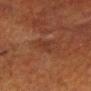Image and clinical context: Automated tile analysis of the lesion measured an area of roughly 3 mm², an outline eccentricity of about 0.8 (0 = round, 1 = elongated), and a symmetry-axis asymmetry near 0.45. And it measured an average lesion color of about L≈28 a*≈19 b*≈25 (CIELAB) and a lesion–skin lightness drop of about 4. Imaged with cross-polarized lighting. The lesion is on the head or neck. This image is a 15 mm lesion crop taken from a total-body photograph. A male patient, about 65 years old.